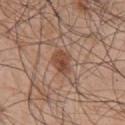Notes:
- workup · catalogued during a skin exam; not biopsied
- site · the chest
- subject · male, in their mid-40s
- image-analysis metrics · a lesion color around L≈47 a*≈21 b*≈29 in CIELAB, a lesion–skin lightness drop of about 11, and a lesion-to-skin contrast of about 8 (normalized; higher = more distinct); a border-irregularity rating of about 2/10, internal color variation of about 4 on a 0–10 scale, and radial color variation of about 1
- diameter · ≈3 mm
- imaging modality · 15 mm crop, total-body photography
- lighting · white-light illumination